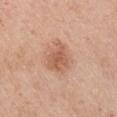notes = total-body-photography surveillance lesion; no biopsy | subject = male, aged 73–77 | lesion diameter = ≈4.5 mm | body site = the arm | lighting = white-light illumination | imaging modality = ~15 mm crop, total-body skin-cancer survey.